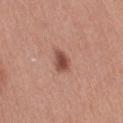Captured during whole-body skin photography for melanoma surveillance; the lesion was not biopsied. A female patient aged 58–62. From the right thigh. A roughly 15 mm field-of-view crop from a total-body skin photograph.